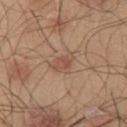On the chest.
Imaged with white-light lighting.
A region of skin cropped from a whole-body photographic capture, roughly 15 mm wide.
A male patient in their mid-40s.